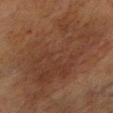Case summary:
* notes: no biopsy performed (imaged during a skin exam)
* tile lighting: cross-polarized illumination
* patient: male, aged around 70
* lesion diameter: about 12 mm
* image: ~15 mm crop, total-body skin-cancer survey
* site: the right lower leg